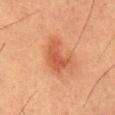notes — imaged on a skin check; not biopsied | body site — the abdomen | patient — male, aged 53 to 57 | image source — ~15 mm tile from a whole-body skin photo.